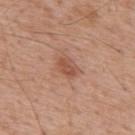| key | value |
|---|---|
| workup | total-body-photography surveillance lesion; no biopsy |
| patient | male, in their 60s |
| TBP lesion metrics | a lesion color around L≈52 a*≈24 b*≈30 in CIELAB and a normalized lesion–skin contrast near 6.5; a border-irregularity rating of about 2/10, a color-variation rating of about 2.5/10, and a peripheral color-asymmetry measure near 1 |
| imaging modality | ~15 mm tile from a whole-body skin photo |
| site | the mid back |
| size | ≈2.5 mm |